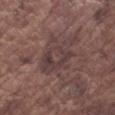Findings:
• biopsy status · no biopsy performed (imaged during a skin exam)
• site · the left forearm
• illumination · white-light illumination
• image · ~15 mm tile from a whole-body skin photo
• lesion size · ≈6 mm
• patient · male, aged 73–77